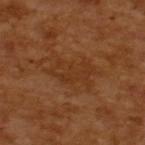biopsy_status: not biopsied; imaged during a skin examination
lighting: cross-polarized
image:
  source: total-body photography crop
  field_of_view_mm: 15
patient:
  sex: male
  age_approx: 65
lesion_size:
  long_diameter_mm_approx: 5.0